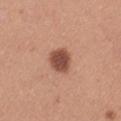Q: Is there a histopathology result?
A: imaged on a skin check; not biopsied
Q: Lesion location?
A: the left thigh
Q: What kind of image is this?
A: ~15 mm tile from a whole-body skin photo
Q: What is the lesion's diameter?
A: ~3.5 mm (longest diameter)
Q: What did automated image analysis measure?
A: an eccentricity of roughly 0.65; an average lesion color of about L≈49 a*≈24 b*≈29 (CIELAB) and a normalized border contrast of about 10.5; a border-irregularity rating of about 1.5/10 and radial color variation of about 0.5
Q: Who is the patient?
A: female, aged approximately 35